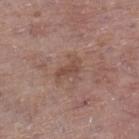The lesion was photographed on a routine skin check and not biopsied; there is no pathology result. The recorded lesion diameter is about 3.5 mm. The subject is a female approximately 85 years of age or older. Located on the leg. This image is a 15 mm lesion crop taken from a total-body photograph. The tile uses white-light illumination.